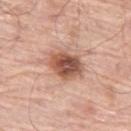  biopsy_status: not biopsied; imaged during a skin examination
  image:
    source: total-body photography crop
    field_of_view_mm: 15
  site: leg
  patient:
    sex: male
    age_approx: 80